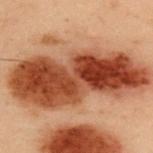workup: total-body-photography surveillance lesion; no biopsy
patient: male, aged around 55
automated lesion analysis: a footprint of about 75 mm²; a lesion color around L≈40 a*≈23 b*≈30 in CIELAB, roughly 16 lightness units darker than nearby skin, and a lesion-to-skin contrast of about 12.5 (normalized; higher = more distinct); border irregularity of about 5 on a 0–10 scale, a within-lesion color-variation index near 9.5/10, and radial color variation of about 4; a classifier nevus-likeness of about 85/100 and a detector confidence of about 100 out of 100 that the crop contains a lesion
body site: the upper back
imaging modality: ~15 mm tile from a whole-body skin photo
diameter: about 15 mm